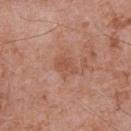Q: Was this lesion biopsied?
A: total-body-photography surveillance lesion; no biopsy
Q: What kind of image is this?
A: 15 mm crop, total-body photography
Q: Who is the patient?
A: male, aged 68 to 72
Q: What is the lesion's diameter?
A: ~2.5 mm (longest diameter)
Q: Where on the body is the lesion?
A: the chest